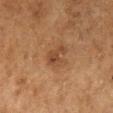This lesion was catalogued during total-body skin photography and was not selected for biopsy.
The lesion is on the right lower leg.
This image is a 15 mm lesion crop taken from a total-body photograph.
The subject is a male about 65 years old.